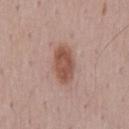Impression:
Imaged during a routine full-body skin examination; the lesion was not biopsied and no histopathology is available.
Image and clinical context:
A male subject roughly 50 years of age. The recorded lesion diameter is about 4.5 mm. The lesion is on the mid back. A 15 mm close-up tile from a total-body photography series done for melanoma screening.